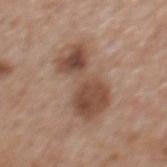Clinical impression:
This lesion was catalogued during total-body skin photography and was not selected for biopsy.
Image and clinical context:
Cropped from a total-body skin-imaging series; the visible field is about 15 mm. The lesion is on the back. Captured under white-light illumination. A male subject in their mid-60s.